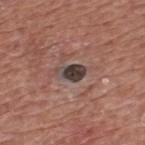Case summary:
* notes — no biopsy performed (imaged during a skin exam)
* diameter — about 3.5 mm
* site — the back
* subject — male, about 75 years old
* acquisition — ~15 mm tile from a whole-body skin photo